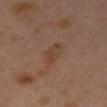Impression:
Captured during whole-body skin photography for melanoma surveillance; the lesion was not biopsied.
Acquisition and patient details:
Located on the left arm. A female patient, aged 38–42. An algorithmic analysis of the crop reported a mean CIELAB color near L≈43 a*≈17 b*≈28, a lesion–skin lightness drop of about 5, and a normalized border contrast of about 5.5. And it measured a classifier nevus-likeness of about 0/100 and lesion-presence confidence of about 100/100. The tile uses cross-polarized illumination. A lesion tile, about 15 mm wide, cut from a 3D total-body photograph. The lesion's longest dimension is about 3.5 mm.